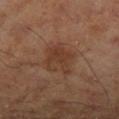Assessment: This lesion was catalogued during total-body skin photography and was not selected for biopsy. Background: A male subject, aged around 45. The tile uses cross-polarized illumination. Measured at roughly 4.5 mm in maximum diameter. Located on the left lower leg. A lesion tile, about 15 mm wide, cut from a 3D total-body photograph. The lesion-visualizer software estimated an average lesion color of about L≈37 a*≈19 b*≈29 (CIELAB), roughly 7 lightness units darker than nearby skin, and a normalized border contrast of about 6.5. The analysis additionally found border irregularity of about 3 on a 0–10 scale and internal color variation of about 3.5 on a 0–10 scale. The analysis additionally found a detector confidence of about 100 out of 100 that the crop contains a lesion.